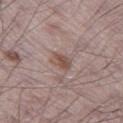Captured during whole-body skin photography for melanoma surveillance; the lesion was not biopsied. This image is a 15 mm lesion crop taken from a total-body photograph. On the left thigh. About 2.5 mm across. A male subject aged around 70.